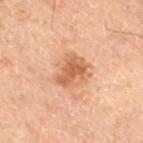follow-up: imaged on a skin check; not biopsied | site: the right thigh | subject: male, roughly 70 years of age | image: ~15 mm tile from a whole-body skin photo | diameter: ≈4.5 mm | tile lighting: cross-polarized illumination | automated metrics: an eccentricity of roughly 0.7; an automated nevus-likeness rating near 30 out of 100 and a detector confidence of about 100 out of 100 that the crop contains a lesion.